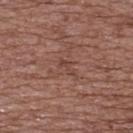Findings:
* follow-up — catalogued during a skin exam; not biopsied
* illumination — white-light illumination
* image source — ~15 mm crop, total-body skin-cancer survey
* subject — female, about 75 years old
* site — the back